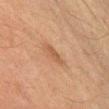Q: What did automated image analysis measure?
A: an eccentricity of roughly 0.85 and a symmetry-axis asymmetry near 0.2; an average lesion color of about L≈42 a*≈18 b*≈28 (CIELAB), roughly 7 lightness units darker than nearby skin, and a normalized border contrast of about 6
Q: What is the imaging modality?
A: ~15 mm crop, total-body skin-cancer survey
Q: Patient demographics?
A: male, roughly 60 years of age
Q: How was the tile lit?
A: cross-polarized illumination
Q: How large is the lesion?
A: ≈3 mm
Q: Where on the body is the lesion?
A: the arm